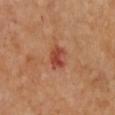Clinical impression: This lesion was catalogued during total-body skin photography and was not selected for biopsy. Clinical summary: A roughly 15 mm field-of-view crop from a total-body skin photograph. An algorithmic analysis of the crop reported an automated nevus-likeness rating near 5 out of 100 and lesion-presence confidence of about 100/100. The lesion is located on the chest. The patient is a female aged 43–47. The tile uses cross-polarized illumination. About 3 mm across.